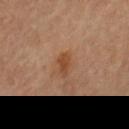{"biopsy_status": "not biopsied; imaged during a skin examination", "image": {"source": "total-body photography crop", "field_of_view_mm": 15}, "lighting": "cross-polarized", "site": "left upper arm", "patient": {"sex": "female", "age_approx": 70}}